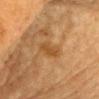<case>
  <biopsy_status>not biopsied; imaged during a skin examination</biopsy_status>
  <lesion_size>
    <long_diameter_mm_approx>3.5</long_diameter_mm_approx>
  </lesion_size>
  <lighting>cross-polarized</lighting>
  <site>chest</site>
  <automated_metrics>
    <cielab_L>44</cielab_L>
    <cielab_a>20</cielab_a>
    <cielab_b>37</cielab_b>
    <color_variation_0_10>1.5</color_variation_0_10>
    <peripheral_color_asymmetry>0.5</peripheral_color_asymmetry>
  </automated_metrics>
  <image>
    <source>total-body photography crop</source>
    <field_of_view_mm>15</field_of_view_mm>
  </image>
  <patient>
    <sex>male</sex>
    <age_approx>85+</age_approx>
  </patient>
</case>